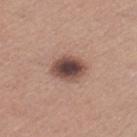<lesion>
  <biopsy_status>not biopsied; imaged during a skin examination</biopsy_status>
  <site>leg</site>
  <lighting>white-light</lighting>
  <image>
    <source>total-body photography crop</source>
    <field_of_view_mm>15</field_of_view_mm>
  </image>
  <lesion_size>
    <long_diameter_mm_approx>4.0</long_diameter_mm_approx>
  </lesion_size>
  <automated_metrics>
    <vs_skin_darker_L>17.0</vs_skin_darker_L>
    <vs_skin_contrast_norm>12.0</vs_skin_contrast_norm>
  </automated_metrics>
  <patient>
    <sex>female</sex>
    <age_approx>55</age_approx>
  </patient>
</lesion>